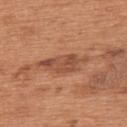This lesion was catalogued during total-body skin photography and was not selected for biopsy. On the upper back. The tile uses white-light illumination. This image is a 15 mm lesion crop taken from a total-body photograph. A male subject, approximately 65 years of age. The total-body-photography lesion software estimated a lesion area of about 9.5 mm², a shape eccentricity near 0.9, and two-axis asymmetry of about 0.35. It also reported a lesion color around L≈50 a*≈24 b*≈33 in CIELAB and a normalized lesion–skin contrast near 7. It also reported border irregularity of about 5 on a 0–10 scale, a within-lesion color-variation index near 3.5/10, and a peripheral color-asymmetry measure near 1. The software also gave a classifier nevus-likeness of about 0/100 and a lesion-detection confidence of about 80/100. About 6 mm across.